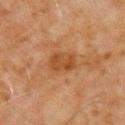follow-up = total-body-photography surveillance lesion; no biopsy | image source = ~15 mm tile from a whole-body skin photo | diameter = ≈3.5 mm | patient = male, aged 68 to 72 | anatomic site = the chest.